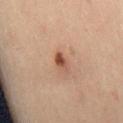Clinical impression: The lesion was photographed on a routine skin check and not biopsied; there is no pathology result. Clinical summary: About 2.5 mm across. The subject is aged 53 to 57. The total-body-photography lesion software estimated a lesion area of about 3 mm², a shape eccentricity near 0.8, and a shape-asymmetry score of about 0.25 (0 = symmetric). The analysis additionally found a border-irregularity index near 2.5/10, internal color variation of about 5 on a 0–10 scale, and a peripheral color-asymmetry measure near 1.5. It also reported a classifier nevus-likeness of about 85/100 and a lesion-detection confidence of about 100/100. The lesion is on the right thigh. The tile uses cross-polarized illumination. This image is a 15 mm lesion crop taken from a total-body photograph.